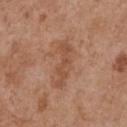The lesion was tiled from a total-body skin photograph and was not biopsied. A female subject, approximately 75 years of age. The lesion is located on the chest. Cropped from a whole-body photographic skin survey; the tile spans about 15 mm. Imaged with white-light lighting.